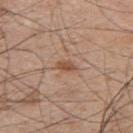<case>
<biopsy_status>not biopsied; imaged during a skin examination</biopsy_status>
<image>
  <source>total-body photography crop</source>
  <field_of_view_mm>15</field_of_view_mm>
</image>
<lesion_size>
  <long_diameter_mm_approx>2.5</long_diameter_mm_approx>
</lesion_size>
<lighting>white-light</lighting>
<site>upper back</site>
<patient>
  <sex>male</sex>
  <age_approx>50</age_approx>
</patient>
</case>